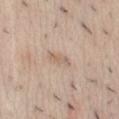Part of a total-body skin-imaging series; this lesion was reviewed on a skin check and was not flagged for biopsy.
Longest diameter approximately 3 mm.
The patient is a female about 40 years old.
The lesion is on the abdomen.
Automated image analysis of the tile measured a footprint of about 2.5 mm², a shape eccentricity near 0.95, and a symmetry-axis asymmetry near 0.35. The analysis additionally found a mean CIELAB color near L≈62 a*≈15 b*≈28, a lesion–skin lightness drop of about 8, and a normalized border contrast of about 5.5.
This image is a 15 mm lesion crop taken from a total-body photograph.